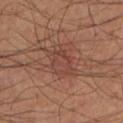follow-up: catalogued during a skin exam; not biopsied | patient: male, aged 48 to 52 | anatomic site: the left lower leg | illumination: cross-polarized | image source: total-body-photography crop, ~15 mm field of view | TBP lesion metrics: a lesion area of about 9.5 mm², an outline eccentricity of about 0.7 (0 = round, 1 = elongated), and a symmetry-axis asymmetry near 0.3; a lesion color around L≈40 a*≈21 b*≈25 in CIELAB and a lesion–skin lightness drop of about 5; an automated nevus-likeness rating near 0 out of 100 and a detector confidence of about 100 out of 100 that the crop contains a lesion | size: ~4 mm (longest diameter).